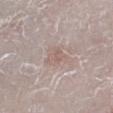{
  "biopsy_status": "not biopsied; imaged during a skin examination",
  "site": "left lower leg",
  "patient": {
    "sex": "male",
    "age_approx": 80
  },
  "lighting": "white-light",
  "image": {
    "source": "total-body photography crop",
    "field_of_view_mm": 15
  }
}